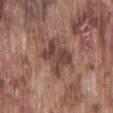follow-up=catalogued during a skin exam; not biopsied
location=the lower back
lighting=white-light
patient=male, aged approximately 75
automated lesion analysis=a border-irregularity rating of about 4/10, a color-variation rating of about 4/10, and peripheral color asymmetry of about 1.5; a classifier nevus-likeness of about 0/100 and lesion-presence confidence of about 100/100
lesion diameter=~4 mm (longest diameter)
image source=15 mm crop, total-body photography